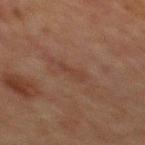Q: Was a biopsy performed?
A: catalogued during a skin exam; not biopsied
Q: Lesion location?
A: the chest
Q: What is the imaging modality?
A: ~15 mm crop, total-body skin-cancer survey
Q: Automated lesion metrics?
A: a footprint of about 2.5 mm², an eccentricity of roughly 0.95, and a shape-asymmetry score of about 0.4 (0 = symmetric); border irregularity of about 4.5 on a 0–10 scale, internal color variation of about 0 on a 0–10 scale, and a peripheral color-asymmetry measure near 0
Q: Who is the patient?
A: male, approximately 65 years of age